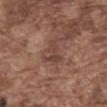Q: Was this lesion biopsied?
A: no biopsy performed (imaged during a skin exam)
Q: What lighting was used for the tile?
A: white-light
Q: What kind of image is this?
A: 15 mm crop, total-body photography
Q: Lesion size?
A: about 3.5 mm
Q: Patient demographics?
A: male, aged approximately 75
Q: What is the anatomic site?
A: the abdomen